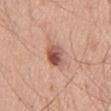Captured during whole-body skin photography for melanoma surveillance; the lesion was not biopsied.